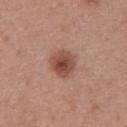Recorded during total-body skin imaging; not selected for excision or biopsy.
The subject is a female about 35 years old.
The total-body-photography lesion software estimated an area of roughly 9 mm², an outline eccentricity of about 0.35 (0 = round, 1 = elongated), and two-axis asymmetry of about 0.1. And it measured a border-irregularity index near 1/10 and radial color variation of about 1.5. It also reported lesion-presence confidence of about 100/100.
On the chest.
Cropped from a total-body skin-imaging series; the visible field is about 15 mm.
This is a white-light tile.